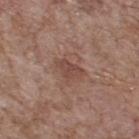Notes:
- workup — total-body-photography surveillance lesion; no biopsy
- acquisition — 15 mm crop, total-body photography
- tile lighting — white-light
- lesion size — ≈3.5 mm
- anatomic site — the upper back
- subject — male, approximately 70 years of age